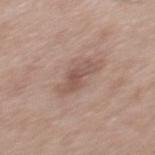* acquisition: 15 mm crop, total-body photography
* patient: male, in their mid-50s
* lighting: white-light
* lesion diameter: about 4 mm
* location: the mid back